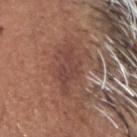Recorded during total-body skin imaging; not selected for excision or biopsy. A male patient, about 75 years old. On the head or neck. A 15 mm crop from a total-body photograph taken for skin-cancer surveillance.